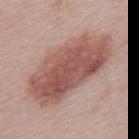Q: Is there a histopathology result?
A: imaged on a skin check; not biopsied